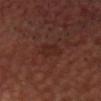This lesion was catalogued during total-body skin photography and was not selected for biopsy. The lesion is on the head or neck. A male subject roughly 55 years of age. Cropped from a whole-body photographic skin survey; the tile spans about 15 mm. The total-body-photography lesion software estimated a classifier nevus-likeness of about 0/100 and a detector confidence of about 100 out of 100 that the crop contains a lesion. Longest diameter approximately 3.5 mm. Imaged with cross-polarized lighting.